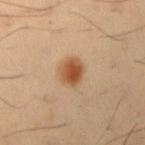| field | value |
|---|---|
| workup | no biopsy performed (imaged during a skin exam) |